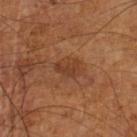Acquisition and patient details:
Automated image analysis of the tile measured a lesion area of about 4.5 mm², an eccentricity of roughly 0.8, and two-axis asymmetry of about 0.25. The analysis additionally found a lesion color around L≈37 a*≈22 b*≈31 in CIELAB, a lesion–skin lightness drop of about 7, and a lesion-to-skin contrast of about 6 (normalized; higher = more distinct). It also reported lesion-presence confidence of about 100/100. The tile uses cross-polarized illumination. The lesion's longest dimension is about 3 mm. The subject is a male in their mid-60s. A 15 mm close-up tile from a total-body photography series done for melanoma screening. The lesion is located on the left lower leg.